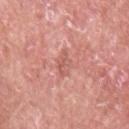No biopsy was performed on this lesion — it was imaged during a full skin examination and was not determined to be concerning. A 15 mm crop from a total-body photograph taken for skin-cancer surveillance. Approximately 3 mm at its widest. The subject is a female aged around 60. The lesion is on the arm.